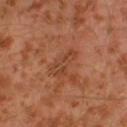Q: Was a biopsy performed?
A: no biopsy performed (imaged during a skin exam)
Q: How was the tile lit?
A: cross-polarized illumination
Q: What are the patient's age and sex?
A: male, about 30 years old
Q: What kind of image is this?
A: total-body-photography crop, ~15 mm field of view
Q: What is the anatomic site?
A: the left leg
Q: What is the lesion's diameter?
A: about 4 mm